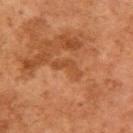Findings:
- workup · catalogued during a skin exam; not biopsied
- image · ~15 mm crop, total-body skin-cancer survey
- anatomic site · the right upper arm
- size · about 4 mm
- lighting · cross-polarized illumination
- subject · female, approximately 60 years of age